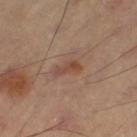Part of a total-body skin-imaging series; this lesion was reviewed on a skin check and was not flagged for biopsy. Imaged with cross-polarized lighting. From the left thigh. Approximately 3 mm at its widest. A roughly 15 mm field-of-view crop from a total-body skin photograph.